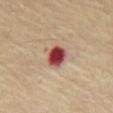Q: Was this lesion biopsied?
A: imaged on a skin check; not biopsied
Q: What is the anatomic site?
A: the chest
Q: Illumination type?
A: cross-polarized illumination
Q: Patient demographics?
A: male, about 70 years old
Q: What is the lesion's diameter?
A: about 3 mm
Q: What kind of image is this?
A: ~15 mm crop, total-body skin-cancer survey